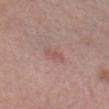| field | value |
|---|---|
| follow-up | no biopsy performed (imaged during a skin exam) |
| diameter | about 3 mm |
| imaging modality | 15 mm crop, total-body photography |
| patient | male, roughly 30 years of age |